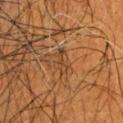This lesion was catalogued during total-body skin photography and was not selected for biopsy.
The tile uses cross-polarized illumination.
A male subject aged around 75.
A roughly 15 mm field-of-view crop from a total-body skin photograph.
Longest diameter approximately 3 mm.
Located on the head or neck.
The total-body-photography lesion software estimated a classifier nevus-likeness of about 0/100.